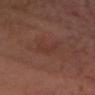biopsy_status: not biopsied; imaged during a skin examination
lighting: cross-polarized
patient:
  sex: male
  age_approx: 55
lesion_size:
  long_diameter_mm_approx: 3.5
image:
  source: total-body photography crop
  field_of_view_mm: 15
site: head or neck